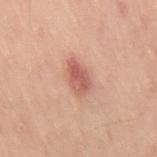Assessment:
Part of a total-body skin-imaging series; this lesion was reviewed on a skin check and was not flagged for biopsy.
Context:
The lesion's longest dimension is about 4 mm. On the right thigh. A 15 mm close-up tile from a total-body photography series done for melanoma screening. A male subject, about 40 years old. The lesion-visualizer software estimated a footprint of about 6 mm², an outline eccentricity of about 0.85 (0 = round, 1 = elongated), and a shape-asymmetry score of about 0.2 (0 = symmetric). The analysis additionally found roughly 10 lightness units darker than nearby skin and a normalized border contrast of about 7.5. The analysis additionally found a border-irregularity rating of about 2/10, a within-lesion color-variation index near 3/10, and peripheral color asymmetry of about 1. And it measured an automated nevus-likeness rating near 85 out of 100. This is a cross-polarized tile.